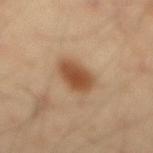Q: Was this lesion biopsied?
A: catalogued during a skin exam; not biopsied
Q: Illumination type?
A: cross-polarized illumination
Q: What kind of image is this?
A: ~15 mm tile from a whole-body skin photo
Q: How large is the lesion?
A: ~4 mm (longest diameter)
Q: Lesion location?
A: the mid back
Q: Patient demographics?
A: male, in their 40s